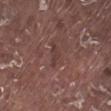Impression: Recorded during total-body skin imaging; not selected for excision or biopsy. Background: A 15 mm crop from a total-body photograph taken for skin-cancer surveillance. The patient is a male in their mid-70s. From the left lower leg.